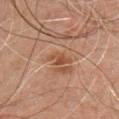Clinical impression:
Part of a total-body skin-imaging series; this lesion was reviewed on a skin check and was not flagged for biopsy.
Background:
A male subject in their mid-40s. This image is a 15 mm lesion crop taken from a total-body photograph. The lesion is on the chest.